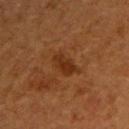Captured during whole-body skin photography for melanoma surveillance; the lesion was not biopsied. Measured at roughly 4 mm in maximum diameter. A female patient, about 40 years old. Located on the upper back. The lesion-visualizer software estimated border irregularity of about 3.5 on a 0–10 scale, internal color variation of about 1.5 on a 0–10 scale, and radial color variation of about 0.5. It also reported lesion-presence confidence of about 100/100. A close-up tile cropped from a whole-body skin photograph, about 15 mm across.